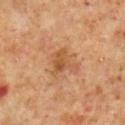{
  "biopsy_status": "not biopsied; imaged during a skin examination",
  "site": "leg",
  "image": {
    "source": "total-body photography crop",
    "field_of_view_mm": 15
  },
  "patient": {
    "sex": "male",
    "age_approx": 60
  }
}